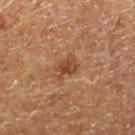Impression:
The lesion was photographed on a routine skin check and not biopsied; there is no pathology result.
Context:
This is a cross-polarized tile. A male patient about 75 years old. Cropped from a whole-body photographic skin survey; the tile spans about 15 mm. Located on the right lower leg.